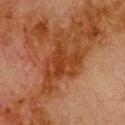notes: no biopsy performed (imaged during a skin exam)
location: the upper back
patient: male, in their 80s
TBP lesion metrics: an area of roughly 13 mm², an outline eccentricity of about 0.7 (0 = round, 1 = elongated), and two-axis asymmetry of about 0.4; a mean CIELAB color near L≈31 a*≈23 b*≈32, roughly 7 lightness units darker than nearby skin, and a lesion-to-skin contrast of about 8 (normalized; higher = more distinct); internal color variation of about 4 on a 0–10 scale and radial color variation of about 1.5
diameter: ~5 mm (longest diameter)
lighting: cross-polarized illumination
image source: total-body-photography crop, ~15 mm field of view